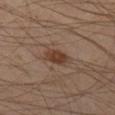Impression: Part of a total-body skin-imaging series; this lesion was reviewed on a skin check and was not flagged for biopsy. Acquisition and patient details: A 15 mm close-up extracted from a 3D total-body photography capture. Automated tile analysis of the lesion measured a border-irregularity index near 2.5/10, a color-variation rating of about 2.5/10, and peripheral color asymmetry of about 1. It also reported a classifier nevus-likeness of about 90/100 and a detector confidence of about 100 out of 100 that the crop contains a lesion. Approximately 3 mm at its widest. This is a cross-polarized tile. The patient is a male roughly 45 years of age. The lesion is located on the left thigh.